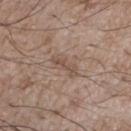Context: A male patient, in their mid- to late 70s. The lesion is on the chest. A 15 mm close-up tile from a total-body photography series done for melanoma screening. The lesion-visualizer software estimated a footprint of about 5.5 mm², an eccentricity of roughly 0.85, and a shape-asymmetry score of about 0.25 (0 = symmetric). It also reported a lesion color around L≈51 a*≈16 b*≈25 in CIELAB and a normalized border contrast of about 5.5. Captured under white-light illumination. Longest diameter approximately 3.5 mm.